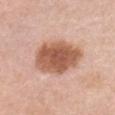Q: Is there a histopathology result?
A: total-body-photography surveillance lesion; no biopsy
Q: What is the imaging modality?
A: 15 mm crop, total-body photography
Q: Patient demographics?
A: female, aged approximately 60
Q: What is the anatomic site?
A: the chest
Q: What lighting was used for the tile?
A: white-light illumination